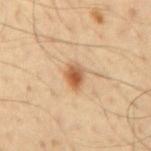site=the mid back | imaging modality=~15 mm tile from a whole-body skin photo | lesion diameter=about 3 mm | illumination=cross-polarized | patient=male, aged 58–62.